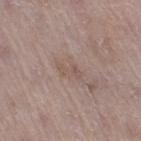Case summary:
- biopsy status — no biopsy performed (imaged during a skin exam)